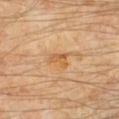The lesion was photographed on a routine skin check and not biopsied; there is no pathology result.
A roughly 15 mm field-of-view crop from a total-body skin photograph.
The lesion-visualizer software estimated internal color variation of about 1 on a 0–10 scale and a peripheral color-asymmetry measure near 0. It also reported an automated nevus-likeness rating near 0 out of 100.
The lesion is on the left lower leg.
The subject is in their mid- to late 50s.